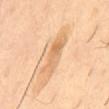workup — catalogued during a skin exam; not biopsied
anatomic site — the abdomen
imaging modality — 15 mm crop, total-body photography
TBP lesion metrics — an area of roughly 10 mm², an outline eccentricity of about 0.95 (0 = round, 1 = elongated), and a shape-asymmetry score of about 0.25 (0 = symmetric); an average lesion color of about L≈68 a*≈19 b*≈39 (CIELAB), roughly 9 lightness units darker than nearby skin, and a lesion-to-skin contrast of about 6 (normalized; higher = more distinct); border irregularity of about 4 on a 0–10 scale, a color-variation rating of about 5/10, and a peripheral color-asymmetry measure near 1.5
tile lighting — cross-polarized
patient — male, about 55 years old